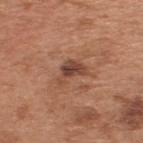Imaged during a routine full-body skin examination; the lesion was not biopsied and no histopathology is available.
The lesion's longest dimension is about 3.5 mm.
A male patient in their mid-60s.
An algorithmic analysis of the crop reported a footprint of about 6 mm², a shape eccentricity near 0.8, and a shape-asymmetry score of about 0.55 (0 = symmetric). The analysis additionally found a lesion color around L≈45 a*≈22 b*≈28 in CIELAB and roughly 11 lightness units darker than nearby skin. And it measured border irregularity of about 5.5 on a 0–10 scale, a color-variation rating of about 4/10, and radial color variation of about 1.
Located on the upper back.
This is a white-light tile.
A roughly 15 mm field-of-view crop from a total-body skin photograph.